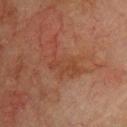Notes:
• follow-up · imaged on a skin check; not biopsied
• image source · ~15 mm crop, total-body skin-cancer survey
• subject · male, in their mid-70s
• site · the front of the torso